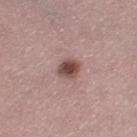Assessment: Part of a total-body skin-imaging series; this lesion was reviewed on a skin check and was not flagged for biopsy. Acquisition and patient details: A female patient, about 50 years old. Imaged with white-light lighting. This image is a 15 mm lesion crop taken from a total-body photograph. The lesion is on the right thigh.